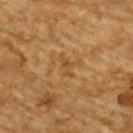<tbp_lesion>
<biopsy_status>not biopsied; imaged during a skin examination</biopsy_status>
<patient>
  <sex>male</sex>
  <age_approx>85+</age_approx>
</patient>
<image>
  <source>total-body photography crop</source>
  <field_of_view_mm>15</field_of_view_mm>
</image>
<site>back</site>
</tbp_lesion>